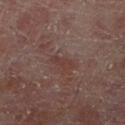follow-up=catalogued during a skin exam; not biopsied | tile lighting=cross-polarized illumination | subject=male, aged around 60 | automated lesion analysis=an average lesion color of about L≈32 a*≈17 b*≈18 (CIELAB), roughly 5 lightness units darker than nearby skin, and a normalized lesion–skin contrast near 5; an automated nevus-likeness rating near 0 out of 100 and a detector confidence of about 95 out of 100 that the crop contains a lesion | lesion size=~3 mm (longest diameter) | image source=15 mm crop, total-body photography | anatomic site=the right lower leg.